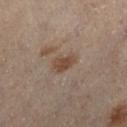  biopsy_status: not biopsied; imaged during a skin examination
  image:
    source: total-body photography crop
    field_of_view_mm: 15
  lighting: cross-polarized
  lesion_size:
    long_diameter_mm_approx: 3.0
  site: right lower leg
  patient:
    sex: male
    age_approx: 55